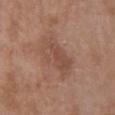Assessment: No biopsy was performed on this lesion — it was imaged during a full skin examination and was not determined to be concerning. Clinical summary: The lesion is located on the chest. This image is a 15 mm lesion crop taken from a total-body photograph. A female subject aged 68–72.